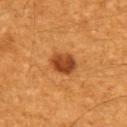Q: Illumination type?
A: cross-polarized
Q: Where on the body is the lesion?
A: the upper back
Q: Automated lesion metrics?
A: a footprint of about 7.5 mm², a shape eccentricity near 0.7, and two-axis asymmetry of about 0.2; a within-lesion color-variation index near 3.5/10 and radial color variation of about 1
Q: Lesion size?
A: ~3.5 mm (longest diameter)
Q: Who is the patient?
A: male, aged 58 to 62
Q: How was this image acquired?
A: total-body-photography crop, ~15 mm field of view